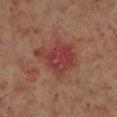No biopsy was performed on this lesion — it was imaged during a full skin examination and was not determined to be concerning. From the left lower leg. Cropped from a whole-body photographic skin survey; the tile spans about 15 mm. A male subject, approximately 60 years of age.